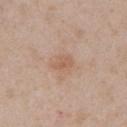notes: no biopsy performed (imaged during a skin exam)
subject: female, aged around 30
image: ~15 mm crop, total-body skin-cancer survey
body site: the left upper arm